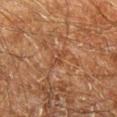Part of a total-body skin-imaging series; this lesion was reviewed on a skin check and was not flagged for biopsy.
A region of skin cropped from a whole-body photographic capture, roughly 15 mm wide.
On the right lower leg.
This is a cross-polarized tile.
A male subject, aged approximately 60.
The lesion's longest dimension is about 3 mm.